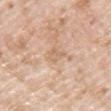<record>
<biopsy_status>not biopsied; imaged during a skin examination</biopsy_status>
<patient>
  <sex>male</sex>
  <age_approx>60</age_approx>
</patient>
<automated_metrics>
  <cielab_L>65</cielab_L>
  <cielab_a>17</cielab_a>
  <cielab_b>33</cielab_b>
  <vs_skin_darker_L>7.0</vs_skin_darker_L>
  <vs_skin_contrast_norm>5.0</vs_skin_contrast_norm>
</automated_metrics>
<image>
  <source>total-body photography crop</source>
  <field_of_view_mm>15</field_of_view_mm>
</image>
<lighting>white-light</lighting>
<site>left upper arm</site>
<lesion_size>
  <long_diameter_mm_approx>3.0</long_diameter_mm_approx>
</lesion_size>
</record>